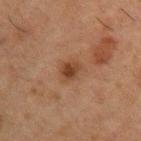Part of a total-body skin-imaging series; this lesion was reviewed on a skin check and was not flagged for biopsy. Imaged with cross-polarized lighting. The total-body-photography lesion software estimated an area of roughly 4.5 mm², an eccentricity of roughly 0.6, and a shape-asymmetry score of about 0.2 (0 = symmetric). And it measured a normalized border contrast of about 8. And it measured a border-irregularity index near 2/10, a color-variation rating of about 3.5/10, and radial color variation of about 1. The software also gave a nevus-likeness score of about 75/100. From the left upper arm. The recorded lesion diameter is about 2.5 mm. A 15 mm close-up extracted from a 3D total-body photography capture. A male patient aged 48–52.